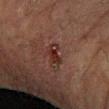<tbp_lesion>
<biopsy_status>not biopsied; imaged during a skin examination</biopsy_status>
<image>
  <source>total-body photography crop</source>
  <field_of_view_mm>15</field_of_view_mm>
</image>
<patient>
  <sex>male</sex>
  <age_approx>50</age_approx>
</patient>
<lighting>cross-polarized</lighting>
<site>left forearm</site>
</tbp_lesion>